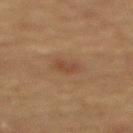Assessment: Recorded during total-body skin imaging; not selected for excision or biopsy. Context: The lesion is located on the mid back. A female patient aged approximately 70. The recorded lesion diameter is about 3 mm. This image is a 15 mm lesion crop taken from a total-body photograph. Imaged with cross-polarized lighting.